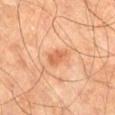{"biopsy_status": "not biopsied; imaged during a skin examination", "automated_metrics": {"area_mm2_approx": 4.5, "eccentricity": 0.85, "shape_asymmetry": 0.25, "cielab_L": 61, "cielab_a": 27, "cielab_b": 39, "border_irregularity_0_10": 2.5, "peripheral_color_asymmetry": 1.0, "nevus_likeness_0_100": 20, "lesion_detection_confidence_0_100": 100}, "site": "lower back", "lighting": "cross-polarized", "lesion_size": {"long_diameter_mm_approx": 3.0}, "image": {"source": "total-body photography crop", "field_of_view_mm": 15}, "patient": {"sex": "male", "age_approx": 60}}